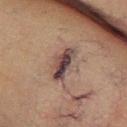Background:
The subject is a male aged 43–47. The lesion is located on the leg. A roughly 15 mm field-of-view crop from a total-body skin photograph. Imaged with cross-polarized lighting.